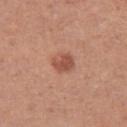| field | value |
|---|---|
| notes | total-body-photography surveillance lesion; no biopsy |
| subject | female, aged 38 to 42 |
| image | total-body-photography crop, ~15 mm field of view |
| site | the leg |